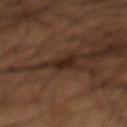<lesion>
  <patient>
    <sex>male</sex>
    <age_approx>55</age_approx>
  </patient>
  <image>
    <source>total-body photography crop</source>
    <field_of_view_mm>15</field_of_view_mm>
  </image>
  <site>abdomen</site>
</lesion>